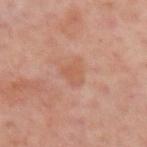{
  "biopsy_status": "not biopsied; imaged during a skin examination",
  "lighting": "cross-polarized",
  "lesion_size": {
    "long_diameter_mm_approx": 3.0
  },
  "site": "left upper arm",
  "patient": {
    "sex": "male",
    "age_approx": 50
  },
  "image": {
    "source": "total-body photography crop",
    "field_of_view_mm": 15
  }
}